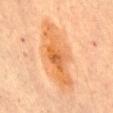biopsy_status: not biopsied; imaged during a skin examination
automated_metrics:
  cielab_L: 60
  cielab_a: 24
  cielab_b: 41
  vs_skin_darker_L: 9.0
  vs_skin_contrast_norm: 7.5
  peripheral_color_asymmetry: 2.0
  lesion_detection_confidence_0_100: 100
image:
  source: total-body photography crop
  field_of_view_mm: 15
patient:
  sex: female
  age_approx: 60
lesion_size:
  long_diameter_mm_approx: 10.5
lighting: cross-polarized
site: abdomen